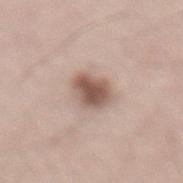Assessment: Recorded during total-body skin imaging; not selected for excision or biopsy. Acquisition and patient details: Automated image analysis of the tile measured a lesion area of about 8.5 mm², a shape eccentricity near 0.65, and a shape-asymmetry score of about 0.15 (0 = symmetric). The analysis additionally found about 15 CIELAB-L* units darker than the surrounding skin. It also reported border irregularity of about 1.5 on a 0–10 scale, a color-variation rating of about 4/10, and peripheral color asymmetry of about 1. The software also gave a nevus-likeness score of about 95/100 and lesion-presence confidence of about 100/100. A male subject, aged 48 to 52. On the lower back. About 3.5 mm across. Cropped from a total-body skin-imaging series; the visible field is about 15 mm. Captured under white-light illumination.